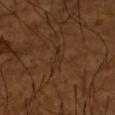Recorded during total-body skin imaging; not selected for excision or biopsy. The recorded lesion diameter is about 2.5 mm. A male subject approximately 65 years of age. A 15 mm close-up tile from a total-body photography series done for melanoma screening. This is a cross-polarized tile. The lesion is located on the right upper arm.